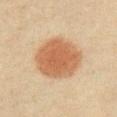{
  "biopsy_status": "not biopsied; imaged during a skin examination",
  "lesion_size": {
    "long_diameter_mm_approx": 5.5
  },
  "patient": {
    "sex": "male",
    "age_approx": 40
  },
  "site": "right upper arm",
  "image": {
    "source": "total-body photography crop",
    "field_of_view_mm": 15
  },
  "lighting": "cross-polarized"
}